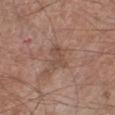The lesion-visualizer software estimated a nevus-likeness score of about 0/100 and lesion-presence confidence of about 100/100. Longest diameter approximately 3.5 mm. Located on the leg. A 15 mm crop from a total-body photograph taken for skin-cancer surveillance. A male patient approximately 60 years of age.